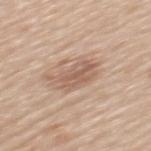Captured during whole-body skin photography for melanoma surveillance; the lesion was not biopsied. The subject is a female aged around 50. The tile uses white-light illumination. The lesion-visualizer software estimated a nevus-likeness score of about 35/100 and lesion-presence confidence of about 100/100. From the upper back. A roughly 15 mm field-of-view crop from a total-body skin photograph.